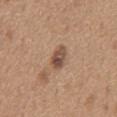This lesion was catalogued during total-body skin photography and was not selected for biopsy. A close-up tile cropped from a whole-body skin photograph, about 15 mm across. The lesion is on the mid back. A male patient, roughly 65 years of age.